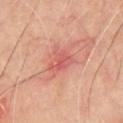Acquisition and patient details: A 15 mm close-up extracted from a 3D total-body photography capture. Imaged with cross-polarized lighting. From the chest. A male subject roughly 65 years of age. Automated tile analysis of the lesion measured a nevus-likeness score of about 0/100 and a detector confidence of about 100 out of 100 that the crop contains a lesion.